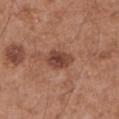Q: Was a biopsy performed?
A: total-body-photography surveillance lesion; no biopsy
Q: What is the lesion's diameter?
A: ≈3.5 mm
Q: Patient demographics?
A: male, aged approximately 55
Q: What is the anatomic site?
A: the arm
Q: What is the imaging modality?
A: 15 mm crop, total-body photography
Q: What lighting was used for the tile?
A: white-light illumination